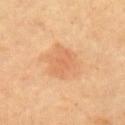Q: Is there a histopathology result?
A: total-body-photography surveillance lesion; no biopsy
Q: Patient demographics?
A: female, aged approximately 70
Q: What kind of image is this?
A: 15 mm crop, total-body photography
Q: Lesion location?
A: the arm
Q: How large is the lesion?
A: about 4 mm